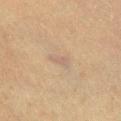<lesion>
<biopsy_status>not biopsied; imaged during a skin examination</biopsy_status>
<patient>
  <sex>male</sex>
  <age_approx>65</age_approx>
</patient>
<site>leg</site>
<lesion_size>
  <long_diameter_mm_approx>2.5</long_diameter_mm_approx>
</lesion_size>
<image>
  <source>total-body photography crop</source>
  <field_of_view_mm>15</field_of_view_mm>
</image>
<lighting>cross-polarized</lighting>
</lesion>